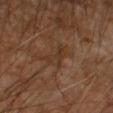No biopsy was performed on this lesion — it was imaged during a full skin examination and was not determined to be concerning. The patient is a male in their mid-50s. This image is a 15 mm lesion crop taken from a total-body photograph. On the left forearm. Imaged with cross-polarized lighting.